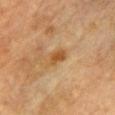biopsy status: total-body-photography surveillance lesion; no biopsy | automated lesion analysis: a footprint of about 3.5 mm², an outline eccentricity of about 0.75 (0 = round, 1 = elongated), and a symmetry-axis asymmetry near 0.25; a border-irregularity rating of about 2/10, a color-variation rating of about 1.5/10, and radial color variation of about 0.5; a nevus-likeness score of about 80/100 and a lesion-detection confidence of about 100/100 | site: the front of the torso | lighting: cross-polarized illumination | lesion diameter: about 2.5 mm | subject: male, aged 83 or over | imaging modality: total-body-photography crop, ~15 mm field of view.